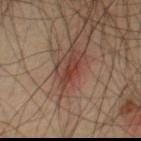{
  "biopsy_status": "not biopsied; imaged during a skin examination",
  "automated_metrics": {
    "area_mm2_approx": 3.5,
    "shape_asymmetry": 0.35,
    "cielab_L": 37,
    "cielab_a": 23,
    "cielab_b": 26,
    "vs_skin_darker_L": 9.0,
    "vs_skin_contrast_norm": 7.5
  },
  "lighting": "cross-polarized",
  "patient": {
    "sex": "male",
    "age_approx": 55
  },
  "site": "chest",
  "image": {
    "source": "total-body photography crop",
    "field_of_view_mm": 15
  }
}